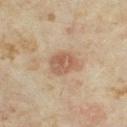Part of a total-body skin-imaging series; this lesion was reviewed on a skin check and was not flagged for biopsy. The lesion's longest dimension is about 3.5 mm. Cropped from a whole-body photographic skin survey; the tile spans about 15 mm. On the right thigh. A female patient about 35 years old.